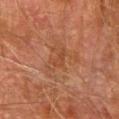{
  "biopsy_status": "not biopsied; imaged during a skin examination",
  "image": {
    "source": "total-body photography crop",
    "field_of_view_mm": 15
  },
  "automated_metrics": {
    "area_mm2_approx": 2.5,
    "shape_asymmetry": 0.45,
    "border_irregularity_0_10": 5.0,
    "color_variation_0_10": 0.0
  },
  "site": "arm",
  "patient": {
    "sex": "male",
    "age_approx": 75
  }
}